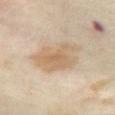Q: What is the anatomic site?
A: the abdomen
Q: Who is the patient?
A: female, in their mid- to late 50s
Q: How was this image acquired?
A: 15 mm crop, total-body photography